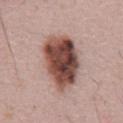notes=no biopsy performed (imaged during a skin exam) | lesion size=about 7 mm | body site=the mid back | illumination=white-light illumination | subject=male, roughly 65 years of age | image source=~15 mm tile from a whole-body skin photo.